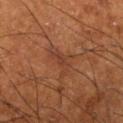Longest diameter approximately 3 mm. On the right lower leg. A close-up tile cropped from a whole-body skin photograph, about 15 mm across. A male patient, in their mid-60s.